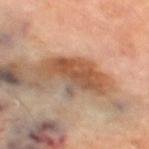Assessment: No biopsy was performed on this lesion — it was imaged during a full skin examination and was not determined to be concerning. Image and clinical context: A male patient, aged approximately 70. The lesion is located on the left thigh. This image is a 15 mm lesion crop taken from a total-body photograph. Automated tile analysis of the lesion measured an area of roughly 17 mm² and a symmetry-axis asymmetry near 0.35. The software also gave a mean CIELAB color near L≈52 a*≈20 b*≈33, a lesion–skin lightness drop of about 12, and a lesion-to-skin contrast of about 8.5 (normalized; higher = more distinct).